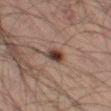Acquisition and patient details:
The subject is a male in their 50s. A 15 mm close-up tile from a total-body photography series done for melanoma screening. Located on the leg. The lesion-visualizer software estimated a footprint of about 4.5 mm² and a shape eccentricity near 0.75. And it measured an average lesion color of about L≈30 a*≈14 b*≈19 (CIELAB), about 11 CIELAB-L* units darker than the surrounding skin, and a normalized border contrast of about 10.5. The analysis additionally found a border-irregularity index near 2/10, a color-variation rating of about 5.5/10, and peripheral color asymmetry of about 1.5. And it measured a classifier nevus-likeness of about 100/100. This is a cross-polarized tile.